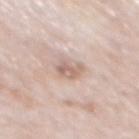Assessment: Recorded during total-body skin imaging; not selected for excision or biopsy. Image and clinical context: The lesion-visualizer software estimated a mean CIELAB color near L≈64 a*≈17 b*≈24, roughly 10 lightness units darker than nearby skin, and a lesion-to-skin contrast of about 6 (normalized; higher = more distinct). The software also gave a border-irregularity rating of about 3/10, a within-lesion color-variation index near 3.5/10, and radial color variation of about 1.5. The software also gave a nevus-likeness score of about 0/100 and lesion-presence confidence of about 100/100. This image is a 15 mm lesion crop taken from a total-body photograph. A male patient, in their 80s. Measured at roughly 3 mm in maximum diameter. From the mid back.